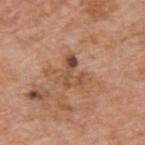Clinical impression: The lesion was photographed on a routine skin check and not biopsied; there is no pathology result. Background: A 15 mm close-up extracted from a 3D total-body photography capture. The tile uses white-light illumination. On the upper back. The subject is a male aged around 60. About 3.5 mm across.